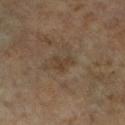workup: catalogued during a skin exam; not biopsied
image: ~15 mm tile from a whole-body skin photo
site: the left leg
patient: female, about 65 years old
tile lighting: cross-polarized illumination
automated metrics: an area of roughly 3 mm² and a shape-asymmetry score of about 0.55 (0 = symmetric); a mean CIELAB color near L≈37 a*≈13 b*≈26, a lesion–skin lightness drop of about 6, and a lesion-to-skin contrast of about 6 (normalized; higher = more distinct); an automated nevus-likeness rating near 0 out of 100